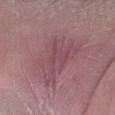Q: Was this lesion biopsied?
A: imaged on a skin check; not biopsied
Q: Who is the patient?
A: male, aged around 65
Q: Illumination type?
A: white-light illumination
Q: Lesion location?
A: the left forearm
Q: What is the imaging modality?
A: ~15 mm tile from a whole-body skin photo